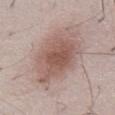Case summary:
• follow-up: catalogued during a skin exam; not biopsied
• image-analysis metrics: a mean CIELAB color near L≈56 a*≈18 b*≈23, a lesion–skin lightness drop of about 11, and a lesion-to-skin contrast of about 8 (normalized; higher = more distinct); an automated nevus-likeness rating near 85 out of 100 and lesion-presence confidence of about 100/100
• illumination: white-light illumination
• location: the abdomen
• patient: male, aged 48 to 52
• imaging modality: 15 mm crop, total-body photography
• diameter: about 8 mm